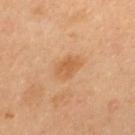{
  "biopsy_status": "not biopsied; imaged during a skin examination",
  "lesion_size": {
    "long_diameter_mm_approx": 3.5
  },
  "patient": {
    "sex": "female",
    "age_approx": 35
  },
  "site": "upper back",
  "image": {
    "source": "total-body photography crop",
    "field_of_view_mm": 15
  }
}